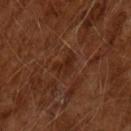Captured under cross-polarized illumination.
The lesion-visualizer software estimated a lesion area of about 5 mm², an outline eccentricity of about 0.75 (0 = round, 1 = elongated), and a shape-asymmetry score of about 0.5 (0 = symmetric). And it measured border irregularity of about 5 on a 0–10 scale, internal color variation of about 2 on a 0–10 scale, and peripheral color asymmetry of about 1. The analysis additionally found an automated nevus-likeness rating near 0 out of 100.
A 15 mm crop from a total-body photograph taken for skin-cancer surveillance.
The subject is a male approximately 65 years of age.
Approximately 3 mm at its widest.